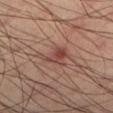Context: This is a cross-polarized tile. A male patient, aged 38 to 42. The lesion is located on the left lower leg. A region of skin cropped from a whole-body photographic capture, roughly 15 mm wide. The lesion-visualizer software estimated a classifier nevus-likeness of about 35/100 and a lesion-detection confidence of about 100/100.